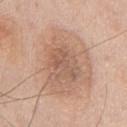No biopsy was performed on this lesion — it was imaged during a full skin examination and was not determined to be concerning. About 4 mm across. Cropped from a total-body skin-imaging series; the visible field is about 15 mm. From the chest. A male patient, aged around 60.